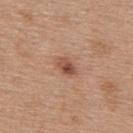Assessment: Captured during whole-body skin photography for melanoma surveillance; the lesion was not biopsied. Background: Cropped from a whole-body photographic skin survey; the tile spans about 15 mm. The lesion is on the upper back. A female subject aged 38–42.